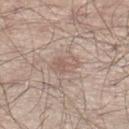Assessment: Part of a total-body skin-imaging series; this lesion was reviewed on a skin check and was not flagged for biopsy. Clinical summary: A male patient aged 53–57. The lesion is on the leg. A close-up tile cropped from a whole-body skin photograph, about 15 mm across. The tile uses white-light illumination. The total-body-photography lesion software estimated an average lesion color of about L≈58 a*≈17 b*≈24 (CIELAB) and a normalized lesion–skin contrast near 5. It also reported border irregularity of about 2 on a 0–10 scale, a color-variation rating of about 3.5/10, and radial color variation of about 1. About 3.5 mm across.